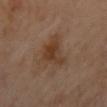Findings:
- follow-up · catalogued during a skin exam; not biopsied
- lighting · cross-polarized
- lesion diameter · ~4.5 mm (longest diameter)
- anatomic site · the chest
- patient · female, approximately 60 years of age
- automated metrics · an outline eccentricity of about 0.45 (0 = round, 1 = elongated) and a symmetry-axis asymmetry near 0.45; a border-irregularity rating of about 5/10, internal color variation of about 4 on a 0–10 scale, and radial color variation of about 1; a classifier nevus-likeness of about 20/100 and a lesion-detection confidence of about 100/100
- acquisition · ~15 mm crop, total-body skin-cancer survey